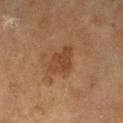Q: Was a biopsy performed?
A: total-body-photography surveillance lesion; no biopsy
Q: Who is the patient?
A: female, roughly 80 years of age
Q: How large is the lesion?
A: about 3.5 mm
Q: Lesion location?
A: the left forearm
Q: Automated lesion metrics?
A: a footprint of about 7.5 mm² and two-axis asymmetry of about 0.3; an average lesion color of about L≈37 a*≈19 b*≈29 (CIELAB), roughly 7 lightness units darker than nearby skin, and a lesion-to-skin contrast of about 6 (normalized; higher = more distinct); a nevus-likeness score of about 5/100 and lesion-presence confidence of about 100/100
Q: How was this image acquired?
A: total-body-photography crop, ~15 mm field of view
Q: Illumination type?
A: cross-polarized illumination